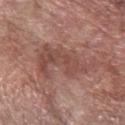Recorded during total-body skin imaging; not selected for excision or biopsy.
Automated tile analysis of the lesion measured an area of roughly 19 mm², an eccentricity of roughly 0.9, and a shape-asymmetry score of about 0.5 (0 = symmetric). And it measured a mean CIELAB color near L≈48 a*≈22 b*≈24 and a normalized border contrast of about 6. It also reported a border-irregularity rating of about 8/10, internal color variation of about 3.5 on a 0–10 scale, and peripheral color asymmetry of about 1.5. The software also gave a classifier nevus-likeness of about 0/100 and a lesion-detection confidence of about 80/100.
This image is a 15 mm lesion crop taken from a total-body photograph.
From the right forearm.
Approximately 8 mm at its widest.
A female patient approximately 60 years of age.